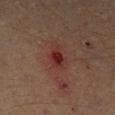{
  "biopsy_status": "not biopsied; imaged during a skin examination",
  "lesion_size": {
    "long_diameter_mm_approx": 4.0
  },
  "lighting": "cross-polarized",
  "patient": {
    "sex": "male",
    "age_approx": 55
  },
  "automated_metrics": {
    "eccentricity": 0.85,
    "shape_asymmetry": 0.2,
    "border_irregularity_0_10": 2.5,
    "peripheral_color_asymmetry": 1.5
  },
  "site": "left lower leg",
  "image": {
    "source": "total-body photography crop",
    "field_of_view_mm": 15
  }
}